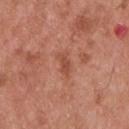notes = no biopsy performed (imaged during a skin exam)
body site = the upper back
lesion size = ~2.5 mm (longest diameter)
illumination = white-light
acquisition = total-body-photography crop, ~15 mm field of view
subject = male, about 55 years old